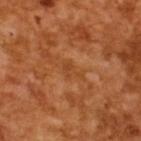Imaged during a routine full-body skin examination; the lesion was not biopsied and no histopathology is available.
The recorded lesion diameter is about 2.5 mm.
This is a cross-polarized tile.
An algorithmic analysis of the crop reported a footprint of about 2.5 mm², an outline eccentricity of about 0.8 (0 = round, 1 = elongated), and a symmetry-axis asymmetry near 0.6.
A roughly 15 mm field-of-view crop from a total-body skin photograph.
A male subject aged 63–67.